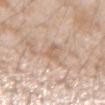<lesion>
<automated_metrics>
  <area_mm2_approx>2.0</area_mm2_approx>
  <eccentricity>0.95</eccentricity>
  <cielab_L>62</cielab_L>
  <cielab_a>17</cielab_a>
  <cielab_b>30</cielab_b>
  <vs_skin_darker_L>7.0</vs_skin_darker_L>
  <vs_skin_contrast_norm>5.0</vs_skin_contrast_norm>
  <border_irregularity_0_10>6.5</border_irregularity_0_10>
  <color_variation_0_10>0.0</color_variation_0_10>
  <peripheral_color_asymmetry>0.0</peripheral_color_asymmetry>
  <nevus_likeness_0_100>0</nevus_likeness_0_100>
  <lesion_detection_confidence_0_100>75</lesion_detection_confidence_0_100>
</automated_metrics>
<lesion_size>
  <long_diameter_mm_approx>3.0</long_diameter_mm_approx>
</lesion_size>
<image>
  <source>total-body photography crop</source>
  <field_of_view_mm>15</field_of_view_mm>
</image>
<patient>
  <sex>male</sex>
  <age_approx>25</age_approx>
</patient>
<lighting>white-light</lighting>
<site>left forearm</site>
</lesion>